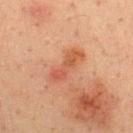Clinical impression:
No biopsy was performed on this lesion — it was imaged during a full skin examination and was not determined to be concerning.
Acquisition and patient details:
Cropped from a total-body skin-imaging series; the visible field is about 15 mm. The lesion is located on the upper back. Approximately 5 mm at its widest. The patient is a male in their mid- to late 30s. Automated image analysis of the tile measured an area of roughly 8 mm², an outline eccentricity of about 0.95 (0 = round, 1 = elongated), and a symmetry-axis asymmetry near 0.3.